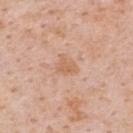<lesion>
  <biopsy_status>not biopsied; imaged during a skin examination</biopsy_status>
  <site>upper back</site>
  <lighting>white-light</lighting>
  <patient>
    <sex>male</sex>
    <age_approx>60</age_approx>
  </patient>
  <lesion_size>
    <long_diameter_mm_approx>2.5</long_diameter_mm_approx>
  </lesion_size>
  <image>
    <source>total-body photography crop</source>
    <field_of_view_mm>15</field_of_view_mm>
  </image>
</lesion>